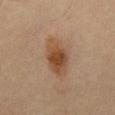Background: About 6 mm across. The patient is a male aged 63 to 67. The tile uses cross-polarized illumination. A close-up tile cropped from a whole-body skin photograph, about 15 mm across. Automated tile analysis of the lesion measured a footprint of about 13 mm², an eccentricity of roughly 0.9, and a symmetry-axis asymmetry near 0.2. And it measured a mean CIELAB color near L≈45 a*≈18 b*≈31 and roughly 10 lightness units darker than nearby skin. The software also gave a border-irregularity rating of about 2.5/10 and a within-lesion color-variation index near 5/10. From the chest.